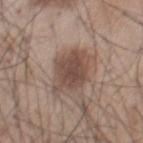biopsy status: imaged on a skin check; not biopsied.